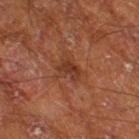Clinical impression:
Imaged during a routine full-body skin examination; the lesion was not biopsied and no histopathology is available.
Clinical summary:
This image is a 15 mm lesion crop taken from a total-body photograph. The subject is a male aged approximately 65. Located on the right thigh.